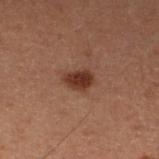No biopsy was performed on this lesion — it was imaged during a full skin examination and was not determined to be concerning.
This is a cross-polarized tile.
A region of skin cropped from a whole-body photographic capture, roughly 15 mm wide.
The subject is a male aged around 60.
The lesion is located on the left lower leg.